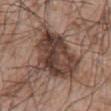Image and clinical context:
A male subject in their mid-60s. A close-up tile cropped from a whole-body skin photograph, about 15 mm across. The lesion is on the arm. Automated tile analysis of the lesion measured an eccentricity of roughly 0.55 and a shape-asymmetry score of about 0.3 (0 = symmetric). The software also gave a normalized border contrast of about 11.5. And it measured a border-irregularity index near 4.5/10 and a color-variation rating of about 8/10. It also reported a lesion-detection confidence of about 100/100. Approximately 7.5 mm at its widest. This is a white-light tile.